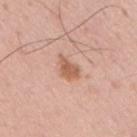| key | value |
|---|---|
| follow-up | no biopsy performed (imaged during a skin exam) |
| image | 15 mm crop, total-body photography |
| location | the back |
| lighting | white-light |
| subject | male, aged approximately 55 |